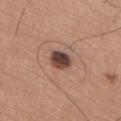follow-up: no biopsy performed (imaged during a skin exam)
patient: male, aged 63–67
tile lighting: white-light
body site: the lower back
TBP lesion metrics: a shape eccentricity near 0.55 and two-axis asymmetry of about 0.15; a nevus-likeness score of about 45/100 and a lesion-detection confidence of about 100/100
imaging modality: 15 mm crop, total-body photography
lesion diameter: ≈3 mm